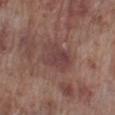biopsy_status: not biopsied; imaged during a skin examination
image:
  source: total-body photography crop
  field_of_view_mm: 15
lighting: white-light
site: right lower leg
automated_metrics:
  area_mm2_approx: 10.0
  shape_asymmetry: 0.2
  cielab_L: 41
  cielab_a: 20
  cielab_b: 20
  vs_skin_darker_L: 7.0
  vs_skin_contrast_norm: 6.5
  peripheral_color_asymmetry: 1.0
patient:
  sex: male
  age_approx: 70
lesion_size:
  long_diameter_mm_approx: 4.0